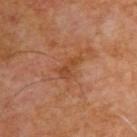follow-up=no biopsy performed (imaged during a skin exam) | TBP lesion metrics=a footprint of about 5 mm² and two-axis asymmetry of about 0.45; an average lesion color of about L≈45 a*≈24 b*≈36 (CIELAB), a lesion–skin lightness drop of about 7, and a normalized lesion–skin contrast near 6; border irregularity of about 5 on a 0–10 scale and a within-lesion color-variation index near 3/10 | subject=male, in their mid- to late 50s | size=about 3.5 mm | illumination=cross-polarized | acquisition=15 mm crop, total-body photography | body site=the upper back.